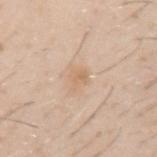follow-up — catalogued during a skin exam; not biopsied
image source — ~15 mm crop, total-body skin-cancer survey
location — the left upper arm
automated metrics — two-axis asymmetry of about 0.35; a nevus-likeness score of about 0/100
illumination — white-light illumination
subject — male, aged approximately 30
lesion size — ~2.5 mm (longest diameter)